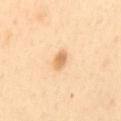Located on the mid back. A female patient aged around 40. A region of skin cropped from a whole-body photographic capture, roughly 15 mm wide.